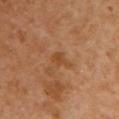<record>
  <biopsy_status>not biopsied; imaged during a skin examination</biopsy_status>
  <patient>
    <sex>female</sex>
    <age_approx>55</age_approx>
  </patient>
  <lesion_size>
    <long_diameter_mm_approx>2.5</long_diameter_mm_approx>
  </lesion_size>
  <site>chest</site>
  <image>
    <source>total-body photography crop</source>
    <field_of_view_mm>15</field_of_view_mm>
  </image>
</record>